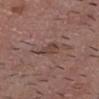Background:
The lesion-visualizer software estimated a lesion area of about 5 mm², an outline eccentricity of about 0.75 (0 = round, 1 = elongated), and a shape-asymmetry score of about 0.4 (0 = symmetric). Located on the head or neck. A 15 mm close-up tile from a total-body photography series done for melanoma screening. A male patient, about 55 years old. The lesion's longest dimension is about 3.5 mm. Captured under white-light illumination.